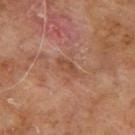| field | value |
|---|---|
| workup | catalogued during a skin exam; not biopsied |
| diameter | about 3 mm |
| site | the upper back |
| patient | male, aged approximately 65 |
| automated lesion analysis | a lesion area of about 3.5 mm², an outline eccentricity of about 0.9 (0 = round, 1 = elongated), and two-axis asymmetry of about 0.25; an average lesion color of about L≈46 a*≈22 b*≈30 (CIELAB), about 7 CIELAB-L* units darker than the surrounding skin, and a normalized lesion–skin contrast near 5.5; a lesion-detection confidence of about 100/100 |
| illumination | cross-polarized illumination |
| image | ~15 mm tile from a whole-body skin photo |